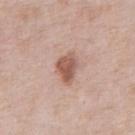Assessment:
The lesion was photographed on a routine skin check and not biopsied; there is no pathology result.
Image and clinical context:
Automated tile analysis of the lesion measured an area of roughly 6.5 mm², an outline eccentricity of about 0.75 (0 = round, 1 = elongated), and a symmetry-axis asymmetry near 0.3. Imaged with white-light lighting. A 15 mm close-up tile from a total-body photography series done for melanoma screening. A male patient in their 80s. Located on the front of the torso. Approximately 3.5 mm at its widest.